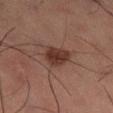| field | value |
|---|---|
| workup | total-body-photography surveillance lesion; no biopsy |
| diameter | about 3.5 mm |
| image | ~15 mm tile from a whole-body skin photo |
| tile lighting | cross-polarized |
| patient | male, aged 58–62 |